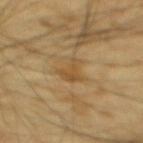Assessment: This lesion was catalogued during total-body skin photography and was not selected for biopsy. Acquisition and patient details: The tile uses cross-polarized illumination. The patient is a male aged 63 to 67. From the back. An algorithmic analysis of the crop reported a lesion color around L≈51 a*≈16 b*≈40 in CIELAB, a lesion–skin lightness drop of about 8, and a normalized border contrast of about 6.5. It also reported an automated nevus-likeness rating near 0 out of 100. A roughly 15 mm field-of-view crop from a total-body skin photograph. Longest diameter approximately 3 mm.